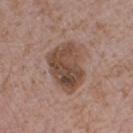On the right forearm.
Captured under white-light illumination.
A male subject, in their mid-70s.
The lesion-visualizer software estimated an area of roughly 19 mm², an outline eccentricity of about 0.7 (0 = round, 1 = elongated), and a symmetry-axis asymmetry near 0.2. And it measured a border-irregularity rating of about 2.5/10 and a color-variation rating of about 6/10.
Approximately 6 mm at its widest.
A 15 mm close-up tile from a total-body photography series done for melanoma screening.